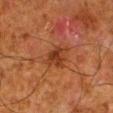Impression:
Captured during whole-body skin photography for melanoma surveillance; the lesion was not biopsied.
Image and clinical context:
This is a cross-polarized tile. A male patient aged 78 to 82. The lesion is located on the right lower leg. The lesion's longest dimension is about 3.5 mm. A region of skin cropped from a whole-body photographic capture, roughly 15 mm wide.